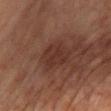biopsy_status: not biopsied; imaged during a skin examination
automated_metrics:
  area_mm2_approx: 9.5
  eccentricity: 0.7
  shape_asymmetry: 0.25
  nevus_likeness_0_100: 5
  lesion_detection_confidence_0_100: 100
patient:
  sex: male
  age_approx: 75
image:
  source: total-body photography crop
  field_of_view_mm: 15
site: chest
lesion_size:
  long_diameter_mm_approx: 4.0